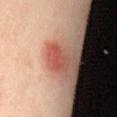Recorded during total-body skin imaging; not selected for excision or biopsy. On the mid back. The patient is a female aged 38 to 42. A lesion tile, about 15 mm wide, cut from a 3D total-body photograph.